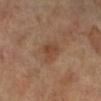Clinical impression: The lesion was tiled from a total-body skin photograph and was not biopsied. Clinical summary: Imaged with cross-polarized lighting. The subject is a female aged 63 to 67. From the left leg. Cropped from a whole-body photographic skin survey; the tile spans about 15 mm.